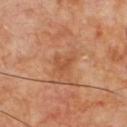Clinical impression:
No biopsy was performed on this lesion — it was imaged during a full skin examination and was not determined to be concerning.
Clinical summary:
A male subject aged around 65. On the chest. Cropped from a whole-body photographic skin survey; the tile spans about 15 mm.